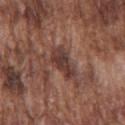workup: no biopsy performed (imaged during a skin exam).